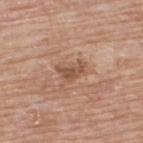Q: Was a biopsy performed?
A: total-body-photography surveillance lesion; no biopsy
Q: Illumination type?
A: white-light
Q: What is the anatomic site?
A: the upper back
Q: What are the patient's age and sex?
A: male, aged 63–67
Q: How was this image acquired?
A: ~15 mm tile from a whole-body skin photo
Q: How large is the lesion?
A: about 3 mm